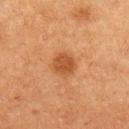Q: Patient demographics?
A: male, aged approximately 75
Q: Automated lesion metrics?
A: a mean CIELAB color near L≈41 a*≈23 b*≈34, a lesion–skin lightness drop of about 9, and a normalized lesion–skin contrast near 7.5; an automated nevus-likeness rating near 90 out of 100 and a lesion-detection confidence of about 100/100
Q: Lesion location?
A: the arm
Q: Illumination type?
A: cross-polarized
Q: How was this image acquired?
A: ~15 mm tile from a whole-body skin photo